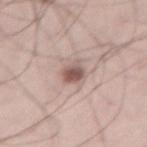<record>
<biopsy_status>not biopsied; imaged during a skin examination</biopsy_status>
<image>
  <source>total-body photography crop</source>
  <field_of_view_mm>15</field_of_view_mm>
</image>
<lighting>white-light</lighting>
<patient>
  <sex>male</sex>
  <age_approx>40</age_approx>
</patient>
<automated_metrics>
  <cielab_L>55</cielab_L>
  <cielab_a>19</cielab_a>
  <cielab_b>21</cielab_b>
  <vs_skin_contrast_norm>9.0</vs_skin_contrast_norm>
</automated_metrics>
<site>right thigh</site>
</record>